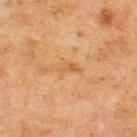Impression:
The lesion was photographed on a routine skin check and not biopsied; there is no pathology result.
Acquisition and patient details:
The lesion is on the upper back. Imaged with cross-polarized lighting. Approximately 3 mm at its widest. A 15 mm crop from a total-body photograph taken for skin-cancer surveillance. A male patient, about 70 years old.